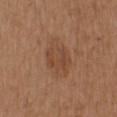notes: total-body-photography surveillance lesion; no biopsy | image: total-body-photography crop, ~15 mm field of view | location: the upper back | subject: female, aged 38 to 42.